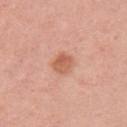Clinical impression: Part of a total-body skin-imaging series; this lesion was reviewed on a skin check and was not flagged for biopsy. Context: Measured at roughly 2.5 mm in maximum diameter. A lesion tile, about 15 mm wide, cut from a 3D total-body photograph. Located on the right upper arm. This is a white-light tile. A female patient aged around 35.